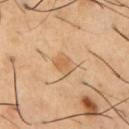Q: Is there a histopathology result?
A: total-body-photography surveillance lesion; no biopsy
Q: Who is the patient?
A: male, approximately 55 years of age
Q: What is the anatomic site?
A: the chest
Q: How was this image acquired?
A: 15 mm crop, total-body photography
Q: What did automated image analysis measure?
A: a footprint of about 5 mm² and an outline eccentricity of about 0.65 (0 = round, 1 = elongated); a lesion color around L≈60 a*≈18 b*≈38 in CIELAB; a detector confidence of about 100 out of 100 that the crop contains a lesion
Q: Illumination type?
A: cross-polarized illumination